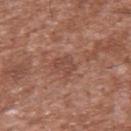No biopsy was performed on this lesion — it was imaged during a full skin examination and was not determined to be concerning. The lesion is located on the back. The tile uses white-light illumination. The patient is a male roughly 45 years of age. A 15 mm close-up extracted from a 3D total-body photography capture. Longest diameter approximately 3 mm.